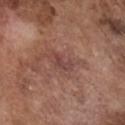Case summary:
- workup: catalogued during a skin exam; not biopsied
- lighting: white-light illumination
- image source: 15 mm crop, total-body photography
- body site: the chest
- TBP lesion metrics: a border-irregularity rating of about 6/10, a within-lesion color-variation index near 0/10, and peripheral color asymmetry of about 0; an automated nevus-likeness rating near 0 out of 100 and lesion-presence confidence of about 95/100
- patient: male, aged around 75
- diameter: ~3 mm (longest diameter)